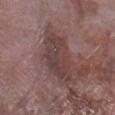The lesion was tiled from a total-body skin photograph and was not biopsied. A male subject aged approximately 75. Measured at roughly 7 mm in maximum diameter. The tile uses white-light illumination. This image is a 15 mm lesion crop taken from a total-body photograph. The lesion is located on the right lower leg.